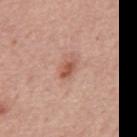Clinical impression: This lesion was catalogued during total-body skin photography and was not selected for biopsy.